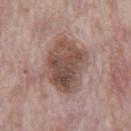Impression:
The lesion was photographed on a routine skin check and not biopsied; there is no pathology result.
Image and clinical context:
A male patient aged around 70. A region of skin cropped from a whole-body photographic capture, roughly 15 mm wide. Located on the abdomen.